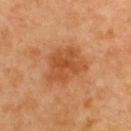{"biopsy_status": "not biopsied; imaged during a skin examination"}